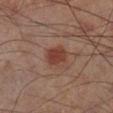No biopsy was performed on this lesion — it was imaged during a full skin examination and was not determined to be concerning. A 15 mm close-up tile from a total-body photography series done for melanoma screening. The lesion is located on the right lower leg. A male subject, in their mid- to late 60s. Measured at roughly 3 mm in maximum diameter. Captured under cross-polarized illumination.